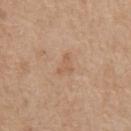notes: no biopsy performed (imaged during a skin exam); image source: ~15 mm crop, total-body skin-cancer survey; tile lighting: white-light; body site: the chest; lesion size: ≈2.5 mm; subject: male, about 70 years old; automated lesion analysis: a border-irregularity index near 6/10, a color-variation rating of about 0/10, and peripheral color asymmetry of about 0.